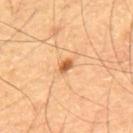Impression: This lesion was catalogued during total-body skin photography and was not selected for biopsy. Clinical summary: The subject is a male about 55 years old. A 15 mm crop from a total-body photograph taken for skin-cancer surveillance. The lesion is on the mid back.